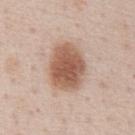Impression:
The lesion was photographed on a routine skin check and not biopsied; there is no pathology result.
Clinical summary:
A region of skin cropped from a whole-body photographic capture, roughly 15 mm wide. The lesion is located on the abdomen. A male patient aged 58 to 62.